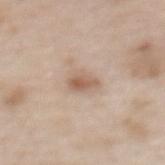notes — no biopsy performed (imaged during a skin exam)
subject — female, roughly 50 years of age
location — the back
image source — total-body-photography crop, ~15 mm field of view